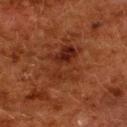Impression:
Recorded during total-body skin imaging; not selected for excision or biopsy.
Clinical summary:
The lesion is located on the back. A 15 mm close-up extracted from a 3D total-body photography capture. A female patient, aged approximately 50.